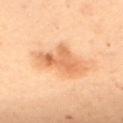{"biopsy_status": "not biopsied; imaged during a skin examination", "image": {"source": "total-body photography crop", "field_of_view_mm": 15}, "lesion_size": {"long_diameter_mm_approx": 5.5}, "site": "back", "lighting": "cross-polarized", "patient": {"sex": "female", "age_approx": 50}}